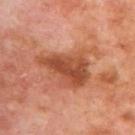Impression: The lesion was photographed on a routine skin check and not biopsied; there is no pathology result. Image and clinical context: Measured at roughly 7.5 mm in maximum diameter. The lesion is located on the back. This image is a 15 mm lesion crop taken from a total-body photograph. A male patient aged around 70.